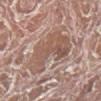Findings:
* follow-up — catalogued during a skin exam; not biopsied
* site — the right lower leg
* lighting — white-light
* diameter — about 6 mm
* imaging modality — ~15 mm tile from a whole-body skin photo
* TBP lesion metrics — a shape eccentricity near 0.5 and a symmetry-axis asymmetry near 0.45; border irregularity of about 6 on a 0–10 scale, a within-lesion color-variation index near 4.5/10, and peripheral color asymmetry of about 1.5
* patient — male, aged 73 to 77